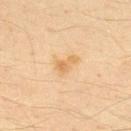notes = imaged on a skin check; not biopsied
location = the upper back
lighting = cross-polarized
subject = male, aged around 35
imaging modality = total-body-photography crop, ~15 mm field of view